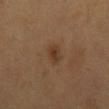Part of a total-body skin-imaging series; this lesion was reviewed on a skin check and was not flagged for biopsy.
A 15 mm close-up extracted from a 3D total-body photography capture.
From the mid back.
A male subject aged approximately 60.